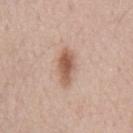Impression:
Part of a total-body skin-imaging series; this lesion was reviewed on a skin check and was not flagged for biopsy.
Image and clinical context:
Imaged with white-light lighting. A lesion tile, about 15 mm wide, cut from a 3D total-body photograph. A male patient approximately 55 years of age. Approximately 4 mm at its widest.